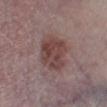Recorded during total-body skin imaging; not selected for excision or biopsy. A male subject, approximately 65 years of age. Captured under white-light illumination. An algorithmic analysis of the crop reported a color-variation rating of about 4.5/10 and a peripheral color-asymmetry measure near 1.5. The analysis additionally found a classifier nevus-likeness of about 80/100 and a detector confidence of about 100 out of 100 that the crop contains a lesion. This image is a 15 mm lesion crop taken from a total-body photograph. Measured at roughly 4.5 mm in maximum diameter. The lesion is on the right lower leg.